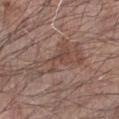This lesion was catalogued during total-body skin photography and was not selected for biopsy. Cropped from a whole-body photographic skin survey; the tile spans about 15 mm. The lesion-visualizer software estimated a lesion color around L≈47 a*≈17 b*≈24 in CIELAB, a lesion–skin lightness drop of about 7, and a lesion-to-skin contrast of about 5.5 (normalized; higher = more distinct). From the left forearm. Captured under white-light illumination. A male subject, roughly 70 years of age.